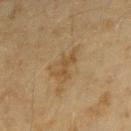Case summary:
* notes · catalogued during a skin exam; not biopsied
* imaging modality · ~15 mm tile from a whole-body skin photo
* image-analysis metrics · a lesion area of about 5.5 mm², an eccentricity of roughly 0.85, and a shape-asymmetry score of about 0.4 (0 = symmetric); an average lesion color of about L≈44 a*≈13 b*≈33 (CIELAB), roughly 6 lightness units darker than nearby skin, and a normalized lesion–skin contrast near 6; a border-irregularity index near 5/10 and internal color variation of about 1.5 on a 0–10 scale; a nevus-likeness score of about 0/100
* subject · female, about 55 years old
* site · the right forearm
* tile lighting · cross-polarized illumination
* diameter · ≈4 mm